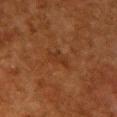Notes:
- biopsy status: total-body-photography surveillance lesion; no biopsy
- acquisition: 15 mm crop, total-body photography
- location: the chest
- patient: female, aged 48–52